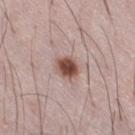Notes:
- biopsy status — no biopsy performed (imaged during a skin exam)
- size — ~3 mm (longest diameter)
- anatomic site — the right thigh
- patient — male, aged approximately 50
- imaging modality — ~15 mm crop, total-body skin-cancer survey
- tile lighting — white-light illumination